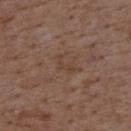The lesion was tiled from a total-body skin photograph and was not biopsied. On the upper back. The subject is a male approximately 50 years of age. A roughly 15 mm field-of-view crop from a total-body skin photograph.